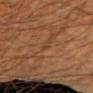No biopsy was performed on this lesion — it was imaged during a full skin examination and was not determined to be concerning. Imaged with cross-polarized lighting. The patient is a male aged 58–62. From the right upper arm. A 15 mm close-up tile from a total-body photography series done for melanoma screening. The recorded lesion diameter is about 1 mm.